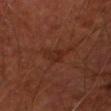notes — catalogued during a skin exam; not biopsied | diameter — ~3 mm (longest diameter) | anatomic site — the left forearm | subject — male, approximately 65 years of age | automated lesion analysis — a lesion area of about 4.5 mm² and a symmetry-axis asymmetry near 0.35; a lesion-to-skin contrast of about 5.5 (normalized; higher = more distinct) | tile lighting — cross-polarized | imaging modality — 15 mm crop, total-body photography.